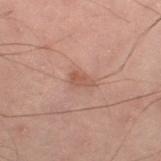The lesion was tiled from a total-body skin photograph and was not biopsied. A lesion tile, about 15 mm wide, cut from a 3D total-body photograph. The subject is a male approximately 50 years of age. Imaged with cross-polarized lighting. Approximately 2.5 mm at its widest. From the left leg.